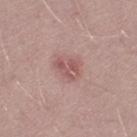notes: total-body-photography surveillance lesion; no biopsy | image-analysis metrics: a mean CIELAB color near L≈54 a*≈23 b*≈20 and about 9 CIELAB-L* units darker than the surrounding skin; a border-irregularity rating of about 4.5/10, internal color variation of about 4.5 on a 0–10 scale, and a peripheral color-asymmetry measure near 2 | patient: male, about 30 years old | imaging modality: ~15 mm tile from a whole-body skin photo | lesion size: ~3 mm (longest diameter).